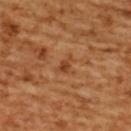| feature | finding |
|---|---|
| workup | no biopsy performed (imaged during a skin exam) |
| illumination | cross-polarized illumination |
| location | the upper back |
| acquisition | total-body-photography crop, ~15 mm field of view |
| patient | female, aged around 55 |